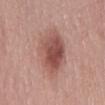Impression:
No biopsy was performed on this lesion — it was imaged during a full skin examination and was not determined to be concerning.
Context:
Captured under white-light illumination. A 15 mm close-up extracted from a 3D total-body photography capture. The lesion is on the mid back. The recorded lesion diameter is about 7.5 mm. A male patient, aged 68–72. An algorithmic analysis of the crop reported an automated nevus-likeness rating near 85 out of 100 and a detector confidence of about 100 out of 100 that the crop contains a lesion.